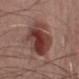Impression:
Captured during whole-body skin photography for melanoma surveillance; the lesion was not biopsied.
Image and clinical context:
Captured under white-light illumination. A male subject aged approximately 65. From the right upper arm. Measured at roughly 9 mm in maximum diameter. Cropped from a whole-body photographic skin survey; the tile spans about 15 mm.